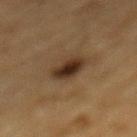The lesion was photographed on a routine skin check and not biopsied; there is no pathology result. On the mid back. A male subject, aged around 85 or older. Cropped from a total-body skin-imaging series; the visible field is about 15 mm.